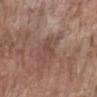notes: no biopsy performed (imaged during a skin exam)
lesion diameter: about 2.5 mm
location: the left forearm
automated lesion analysis: a footprint of about 3.5 mm² and an outline eccentricity of about 0.75 (0 = round, 1 = elongated); an average lesion color of about L≈46 a*≈18 b*≈24 (CIELAB); border irregularity of about 4 on a 0–10 scale, a within-lesion color-variation index near 1.5/10, and a peripheral color-asymmetry measure near 0.5; a classifier nevus-likeness of about 0/100
subject: male, in their 60s
lighting: white-light illumination
image source: ~15 mm tile from a whole-body skin photo